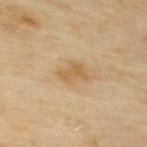The lesion was photographed on a routine skin check and not biopsied; there is no pathology result.
Located on the upper back.
A male subject in their mid-40s.
Automated image analysis of the tile measured an area of roughly 4 mm². And it measured border irregularity of about 3.5 on a 0–10 scale, a within-lesion color-variation index near 1.5/10, and radial color variation of about 0.5. The analysis additionally found a nevus-likeness score of about 0/100.
This image is a 15 mm lesion crop taken from a total-body photograph.
This is a cross-polarized tile.